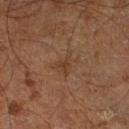Impression: Imaged during a routine full-body skin examination; the lesion was not biopsied and no histopathology is available.